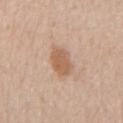biopsy_status: not biopsied; imaged during a skin examination
lighting: white-light
site: chest
image:
  source: total-body photography crop
  field_of_view_mm: 15
automated_metrics:
  eccentricity: 0.75
  shape_asymmetry: 0.2
  border_irregularity_0_10: 2.0
  color_variation_0_10: 2.0
patient:
  sex: male
  age_approx: 65
lesion_size:
  long_diameter_mm_approx: 3.5